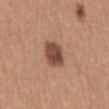Case summary:
• biopsy status: total-body-photography surveillance lesion; no biopsy
• body site: the abdomen
• illumination: white-light
• lesion size: about 3.5 mm
• patient: female, aged around 40
• image: ~15 mm tile from a whole-body skin photo